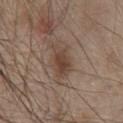This lesion was catalogued during total-body skin photography and was not selected for biopsy.
The subject is a male about 80 years old.
Captured under white-light illumination.
Approximately 4 mm at its widest.
A lesion tile, about 15 mm wide, cut from a 3D total-body photograph.
On the chest.